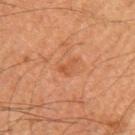Imaged during a routine full-body skin examination; the lesion was not biopsied and no histopathology is available. A male subject aged approximately 65. Cropped from a whole-body photographic skin survey; the tile spans about 15 mm. Automated image analysis of the tile measured an eccentricity of roughly 0.8 and two-axis asymmetry of about 0.55. The software also gave a normalized border contrast of about 5. The software also gave a border-irregularity rating of about 5/10, a within-lesion color-variation index near 1/10, and radial color variation of about 0.5. The analysis additionally found a nevus-likeness score of about 0/100 and a lesion-detection confidence of about 100/100. Located on the arm. Measured at roughly 2.5 mm in maximum diameter. Captured under cross-polarized illumination.